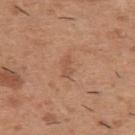follow-up=imaged on a skin check; not biopsied | TBP lesion metrics=a footprint of about 3 mm², an eccentricity of roughly 0.85, and a symmetry-axis asymmetry near 0.35 | imaging modality=15 mm crop, total-body photography | size=≈2.5 mm | illumination=white-light | subject=male, about 40 years old | location=the upper back.